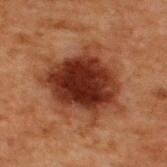No biopsy was performed on this lesion — it was imaged during a full skin examination and was not determined to be concerning.
About 7 mm across.
A male patient aged around 70.
Automated image analysis of the tile measured a border-irregularity rating of about 3.5/10, internal color variation of about 5.5 on a 0–10 scale, and a peripheral color-asymmetry measure near 1.5. The analysis additionally found a classifier nevus-likeness of about 95/100 and a lesion-detection confidence of about 100/100.
From the upper back.
Cropped from a whole-body photographic skin survey; the tile spans about 15 mm.